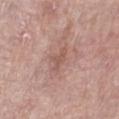follow-up: no biopsy performed (imaged during a skin exam)
subject: female, aged 58 to 62
imaging modality: ~15 mm crop, total-body skin-cancer survey
image-analysis metrics: an area of roughly 4 mm², a shape eccentricity near 0.85, and a shape-asymmetry score of about 0.5 (0 = symmetric); a mean CIELAB color near L≈55 a*≈21 b*≈25 and a normalized border contrast of about 5.5; a nevus-likeness score of about 0/100 and a lesion-detection confidence of about 100/100
anatomic site: the left lower leg
lesion size: ≈3 mm